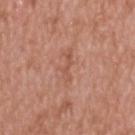Assessment: Captured during whole-body skin photography for melanoma surveillance; the lesion was not biopsied.